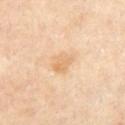Q: Was a biopsy performed?
A: total-body-photography surveillance lesion; no biopsy
Q: What kind of image is this?
A: total-body-photography crop, ~15 mm field of view
Q: Where on the body is the lesion?
A: the abdomen
Q: Who is the patient?
A: female, roughly 50 years of age